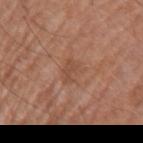Findings:
– notes: catalogued during a skin exam; not biopsied
– patient: male, in their mid- to late 60s
– image source: ~15 mm crop, total-body skin-cancer survey
– body site: the left upper arm